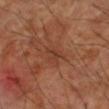{"biopsy_status": "not biopsied; imaged during a skin examination", "lighting": "cross-polarized", "lesion_size": {"long_diameter_mm_approx": 2.5}, "site": "right upper arm", "image": {"source": "total-body photography crop", "field_of_view_mm": 15}, "patient": {"sex": "male", "age_approx": 70}}